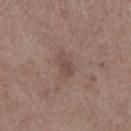Assessment: This lesion was catalogued during total-body skin photography and was not selected for biopsy. Context: A lesion tile, about 15 mm wide, cut from a 3D total-body photograph. The patient is a female aged 63 to 67. From the right lower leg.